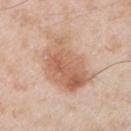Q: How large is the lesion?
A: ≈7 mm
Q: Illumination type?
A: white-light
Q: Patient demographics?
A: male, approximately 55 years of age
Q: Where on the body is the lesion?
A: the arm
Q: What is the imaging modality?
A: total-body-photography crop, ~15 mm field of view